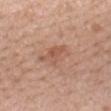Notes:
- follow-up: imaged on a skin check; not biopsied
- automated lesion analysis: an area of roughly 7.5 mm² and two-axis asymmetry of about 0.3; border irregularity of about 3.5 on a 0–10 scale and a peripheral color-asymmetry measure near 1.5; a lesion-detection confidence of about 100/100
- lesion size: ~4.5 mm (longest diameter)
- site: the mid back
- illumination: white-light illumination
- image source: 15 mm crop, total-body photography
- patient: female, aged approximately 50